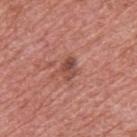This lesion was catalogued during total-body skin photography and was not selected for biopsy. A male patient aged 68 to 72. Located on the upper back. A close-up tile cropped from a whole-body skin photograph, about 15 mm across. Captured under white-light illumination.